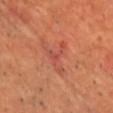workup: total-body-photography surveillance lesion; no biopsy | image-analysis metrics: an area of roughly 7 mm² and a shape-asymmetry score of about 0.8 (0 = symmetric); a mean CIELAB color near L≈51 a*≈31 b*≈32, about 7 CIELAB-L* units darker than the surrounding skin, and a normalized lesion–skin contrast near 5.5; a border-irregularity rating of about 9.5/10, a color-variation rating of about 3/10, and a peripheral color-asymmetry measure near 1 | image source: ~15 mm crop, total-body skin-cancer survey | diameter: about 5 mm | subject: male, aged approximately 65 | site: the front of the torso.